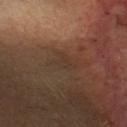Part of a total-body skin-imaging series; this lesion was reviewed on a skin check and was not flagged for biopsy. Cropped from a whole-body photographic skin survey; the tile spans about 15 mm. The lesion is located on the head or neck. This is a cross-polarized tile. The subject is a female roughly 40 years of age. An algorithmic analysis of the crop reported a footprint of about 4.5 mm² and a shape-asymmetry score of about 0.4 (0 = symmetric). The software also gave an average lesion color of about L≈35 a*≈16 b*≈25 (CIELAB) and roughly 4 lightness units darker than nearby skin. It also reported a border-irregularity index near 4.5/10 and a within-lesion color-variation index near 1/10. The analysis additionally found a classifier nevus-likeness of about 0/100 and a lesion-detection confidence of about 60/100.